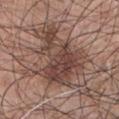Imaged during a routine full-body skin examination; the lesion was not biopsied and no histopathology is available.
A male patient, about 70 years old.
The lesion is located on the chest.
Cropped from a total-body skin-imaging series; the visible field is about 15 mm.
Captured under white-light illumination.
Longest diameter approximately 10 mm.
Automated tile analysis of the lesion measured an area of roughly 39 mm² and an outline eccentricity of about 0.7 (0 = round, 1 = elongated). The software also gave a border-irregularity index near 8/10 and radial color variation of about 3.